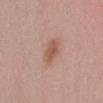{"biopsy_status": "not biopsied; imaged during a skin examination", "lesion_size": {"long_diameter_mm_approx": 3.0}, "automated_metrics": {"cielab_L": 55, "cielab_a": 22, "cielab_b": 27, "vs_skin_contrast_norm": 6.5, "nevus_likeness_0_100": 80}, "lighting": "white-light", "image": {"source": "total-body photography crop", "field_of_view_mm": 15}, "site": "lower back", "patient": {"sex": "female", "age_approx": 25}}